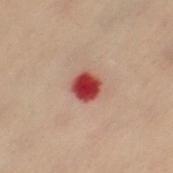workup = no biopsy performed (imaged during a skin exam) | subject = female, aged 58 to 62 | image source = ~15 mm tile from a whole-body skin photo | anatomic site = the right leg | size = about 2.5 mm | tile lighting = cross-polarized | image-analysis metrics = a footprint of about 6.5 mm², an eccentricity of roughly 0.25, and two-axis asymmetry of about 0.2; border irregularity of about 1.5 on a 0–10 scale, internal color variation of about 3 on a 0–10 scale, and radial color variation of about 1.